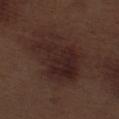| key | value |
|---|---|
| follow-up | total-body-photography surveillance lesion; no biopsy |
| anatomic site | the left thigh |
| patient | male, in their 70s |
| image source | total-body-photography crop, ~15 mm field of view |